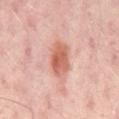Recorded during total-body skin imaging; not selected for excision or biopsy. This is a cross-polarized tile. Approximately 4 mm at its widest. The patient is in their mid-50s. A 15 mm close-up tile from a total-body photography series done for melanoma screening. Located on the mid back.